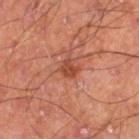Recorded during total-body skin imaging; not selected for excision or biopsy. Imaged with cross-polarized lighting. A 15 mm close-up tile from a total-body photography series done for melanoma screening. About 2 mm across. A male patient, aged 58 to 62. The lesion is located on the left thigh.